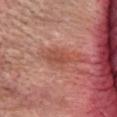Impression: No biopsy was performed on this lesion — it was imaged during a full skin examination and was not determined to be concerning. Background: Automated image analysis of the tile measured a mean CIELAB color near L≈50 a*≈28 b*≈30, about 9 CIELAB-L* units darker than the surrounding skin, and a normalized lesion–skin contrast near 6.5. The software also gave a nevus-likeness score of about 5/100 and a detector confidence of about 100 out of 100 that the crop contains a lesion. The recorded lesion diameter is about 3 mm. The lesion is on the head or neck. A lesion tile, about 15 mm wide, cut from a 3D total-body photograph. A female patient about 70 years old.